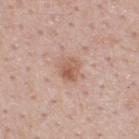The lesion was photographed on a routine skin check and not biopsied; there is no pathology result. A male patient, about 50 years old. This is a white-light tile. Longest diameter approximately 3 mm. From the upper back. A roughly 15 mm field-of-view crop from a total-body skin photograph. The lesion-visualizer software estimated a footprint of about 5.5 mm² and an eccentricity of roughly 0.5. And it measured about 9 CIELAB-L* units darker than the surrounding skin and a normalized border contrast of about 7. The analysis additionally found internal color variation of about 5.5 on a 0–10 scale and radial color variation of about 2. And it measured an automated nevus-likeness rating near 75 out of 100 and lesion-presence confidence of about 100/100.